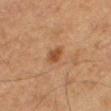biopsy status: total-body-photography surveillance lesion; no biopsy | TBP lesion metrics: a lesion color around L≈39 a*≈19 b*≈30 in CIELAB, about 8 CIELAB-L* units darker than the surrounding skin, and a normalized lesion–skin contrast near 7.5; an automated nevus-likeness rating near 65 out of 100 and lesion-presence confidence of about 100/100 | tile lighting: cross-polarized | image: ~15 mm crop, total-body skin-cancer survey | location: the right thigh | patient: male, about 75 years old | size: about 2.5 mm.